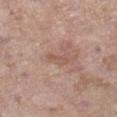  biopsy_status: not biopsied; imaged during a skin examination
  site: right lower leg
  image:
    source: total-body photography crop
    field_of_view_mm: 15
  patient:
    sex: female
    age_approx: 85+